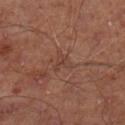Recorded during total-body skin imaging; not selected for excision or biopsy.